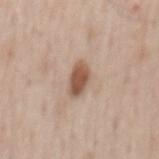Assessment:
Imaged during a routine full-body skin examination; the lesion was not biopsied and no histopathology is available.
Clinical summary:
Longest diameter approximately 3.5 mm. Captured under white-light illumination. On the mid back. A male subject aged 48–52. Cropped from a total-body skin-imaging series; the visible field is about 15 mm.